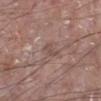A 15 mm close-up extracted from a 3D total-body photography capture.
A male patient aged approximately 65.
Located on the left lower leg.
The tile uses white-light illumination.
The recorded lesion diameter is about 2.5 mm.